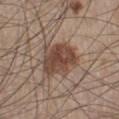No biopsy was performed on this lesion — it was imaged during a full skin examination and was not determined to be concerning. The recorded lesion diameter is about 5 mm. The lesion is located on the leg. The patient is a male aged around 60. Automated image analysis of the tile measured a mean CIELAB color near L≈44 a*≈18 b*≈25 and a lesion-to-skin contrast of about 9.5 (normalized; higher = more distinct). And it measured a border-irregularity index near 2.5/10, internal color variation of about 3.5 on a 0–10 scale, and radial color variation of about 1. The tile uses white-light illumination. A region of skin cropped from a whole-body photographic capture, roughly 15 mm wide.